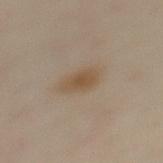Imaged during a routine full-body skin examination; the lesion was not biopsied and no histopathology is available.